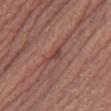<tbp_lesion>
<biopsy_status>not biopsied; imaged during a skin examination</biopsy_status>
<lesion_size>
  <long_diameter_mm_approx>2.5</long_diameter_mm_approx>
</lesion_size>
<image>
  <source>total-body photography crop</source>
  <field_of_view_mm>15</field_of_view_mm>
</image>
<lighting>white-light</lighting>
<patient>
  <sex>female</sex>
  <age_approx>45</age_approx>
</patient>
<site>right lower leg</site>
</tbp_lesion>